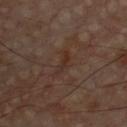biopsy status: no biopsy performed (imaged during a skin exam) | subject: male, about 60 years old | illumination: cross-polarized illumination | acquisition: 15 mm crop, total-body photography | lesion diameter: ≈3.5 mm | anatomic site: the chest.